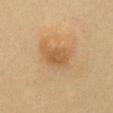Captured during whole-body skin photography for melanoma surveillance; the lesion was not biopsied.
Cropped from a total-body skin-imaging series; the visible field is about 15 mm.
Longest diameter approximately 3.5 mm.
A female patient, in their 60s.
From the chest.